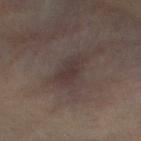The lesion was photographed on a routine skin check and not biopsied; there is no pathology result. This is a cross-polarized tile. The lesion is located on the mid back. A male subject, aged 33–37. A close-up tile cropped from a whole-body skin photograph, about 15 mm across. The lesion-visualizer software estimated an average lesion color of about L≈32 a*≈12 b*≈15 (CIELAB) and a normalized border contrast of about 6.5. Longest diameter approximately 4 mm.